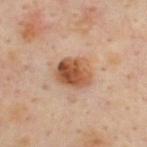biopsy status = total-body-photography surveillance lesion; no biopsy
lesion diameter = ~4 mm (longest diameter)
anatomic site = the upper back
image-analysis metrics = internal color variation of about 8 on a 0–10 scale and radial color variation of about 2.5; an automated nevus-likeness rating near 100 out of 100
imaging modality = ~15 mm tile from a whole-body skin photo
subject = male, roughly 45 years of age
lighting = cross-polarized illumination